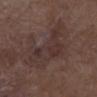{"biopsy_status": "not biopsied; imaged during a skin examination", "image": {"source": "total-body photography crop", "field_of_view_mm": 15}, "lighting": "white-light", "site": "left lower leg", "patient": {"sex": "male", "age_approx": 75}}